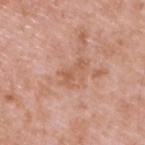{
  "biopsy_status": "not biopsied; imaged during a skin examination",
  "image": {
    "source": "total-body photography crop",
    "field_of_view_mm": 15
  },
  "site": "upper back",
  "patient": {
    "sex": "male",
    "age_approx": 50
  },
  "lighting": "white-light",
  "lesion_size": {
    "long_diameter_mm_approx": 3.0
  }
}